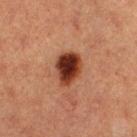A 15 mm close-up tile from a total-body photography series done for melanoma screening. The tile uses cross-polarized illumination. A female subject aged approximately 40. The lesion is on the left thigh. The recorded lesion diameter is about 4 mm. The lesion-visualizer software estimated a lesion area of about 9 mm², a shape eccentricity near 0.65, and a shape-asymmetry score of about 0.15 (0 = symmetric). The analysis additionally found a border-irregularity index near 1.5/10, a color-variation rating of about 5.5/10, and a peripheral color-asymmetry measure near 1.5.